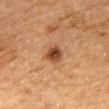Part of a total-body skin-imaging series; this lesion was reviewed on a skin check and was not flagged for biopsy.
A 15 mm close-up extracted from a 3D total-body photography capture.
This is a cross-polarized tile.
A female patient aged around 60.
Approximately 2.5 mm at its widest.
On the mid back.